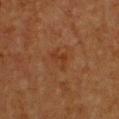This lesion was catalogued during total-body skin photography and was not selected for biopsy. Located on the front of the torso. A region of skin cropped from a whole-body photographic capture, roughly 15 mm wide. This is a cross-polarized tile. Approximately 3 mm at its widest. A female patient about 40 years old.